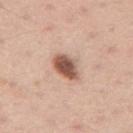<tbp_lesion>
  <image>
    <source>total-body photography crop</source>
    <field_of_view_mm>15</field_of_view_mm>
  </image>
  <patient>
    <sex>male</sex>
    <age_approx>45</age_approx>
  </patient>
  <lesion_size>
    <long_diameter_mm_approx>3.5</long_diameter_mm_approx>
  </lesion_size>
  <site>mid back</site>
  <automated_metrics>
    <eccentricity>0.7</eccentricity>
    <cielab_L>55</cielab_L>
    <cielab_a>21</cielab_a>
    <cielab_b>28</cielab_b>
  </automated_metrics>
</tbp_lesion>